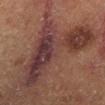<case>
  <biopsy_status>not biopsied; imaged during a skin examination</biopsy_status>
  <site>right lower leg</site>
  <lesion_size>
    <long_diameter_mm_approx>10.5</long_diameter_mm_approx>
  </lesion_size>
  <patient>
    <sex>male</sex>
    <age_approx>55</age_approx>
  </patient>
  <image>
    <source>total-body photography crop</source>
    <field_of_view_mm>15</field_of_view_mm>
  </image>
  <automated_metrics>
    <area_mm2_approx>42.0</area_mm2_approx>
    <eccentricity>0.75</eccentricity>
    <shape_asymmetry>0.75</shape_asymmetry>
    <border_irregularity_0_10>10.0</border_irregularity_0_10>
    <color_variation_0_10>7.0</color_variation_0_10>
    <peripheral_color_asymmetry>2.5</peripheral_color_asymmetry>
    <nevus_likeness_0_100>0</nevus_likeness_0_100>
    <lesion_detection_confidence_0_100>90</lesion_detection_confidence_0_100>
  </automated_metrics>
</case>